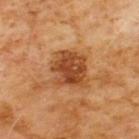Impression:
The lesion was photographed on a routine skin check and not biopsied; there is no pathology result.
Background:
The tile uses cross-polarized illumination. A close-up tile cropped from a whole-body skin photograph, about 15 mm across. The patient is a male approximately 60 years of age. From the chest. The lesion's longest dimension is about 4.5 mm.